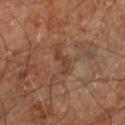The subject is a male aged around 60. Longest diameter approximately 3 mm. A lesion tile, about 15 mm wide, cut from a 3D total-body photograph. On the right leg. The tile uses cross-polarized illumination.